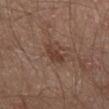Assessment:
The lesion was tiled from a total-body skin photograph and was not biopsied.
Image and clinical context:
A 15 mm close-up extracted from a 3D total-body photography capture. Automated tile analysis of the lesion measured an area of roughly 4.5 mm², a shape eccentricity near 0.85, and a shape-asymmetry score of about 0.3 (0 = symmetric). It also reported an average lesion color of about L≈37 a*≈18 b*≈24 (CIELAB) and about 8 CIELAB-L* units darker than the surrounding skin. Approximately 3 mm at its widest. The lesion is on the right thigh. A male patient approximately 65 years of age.